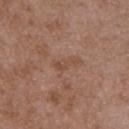The lesion was photographed on a routine skin check and not biopsied; there is no pathology result.
The lesion is located on the upper back.
A 15 mm crop from a total-body photograph taken for skin-cancer surveillance.
Approximately 3 mm at its widest.
A male patient, approximately 50 years of age.